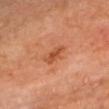Q: Was a biopsy performed?
A: total-body-photography surveillance lesion; no biopsy
Q: Lesion location?
A: the chest
Q: What is the lesion's diameter?
A: ≈3.5 mm
Q: Who is the patient?
A: male, approximately 70 years of age
Q: How was this image acquired?
A: ~15 mm tile from a whole-body skin photo